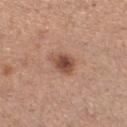follow-up — catalogued during a skin exam; not biopsied | tile lighting — white-light illumination | patient — female, roughly 30 years of age | acquisition — total-body-photography crop, ~15 mm field of view | anatomic site — the left lower leg | lesion diameter — about 3.5 mm.